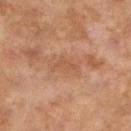Q: Was this lesion biopsied?
A: total-body-photography surveillance lesion; no biopsy
Q: What did automated image analysis measure?
A: a footprint of about 3 mm², an outline eccentricity of about 0.9 (0 = round, 1 = elongated), and a symmetry-axis asymmetry near 0.6; a lesion color around L≈47 a*≈20 b*≈30 in CIELAB, about 5 CIELAB-L* units darker than the surrounding skin, and a normalized lesion–skin contrast near 4.5; border irregularity of about 6 on a 0–10 scale, internal color variation of about 0 on a 0–10 scale, and radial color variation of about 0
Q: What are the patient's age and sex?
A: female, in their 60s
Q: Where on the body is the lesion?
A: the leg
Q: What is the imaging modality?
A: 15 mm crop, total-body photography
Q: Illumination type?
A: cross-polarized
Q: How large is the lesion?
A: about 3 mm